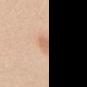  biopsy_status: not biopsied; imaged during a skin examination
  patient:
    sex: female
    age_approx: 20
  automated_metrics:
    eccentricity: 0.8
    shape_asymmetry: 0.3
  lesion_size:
    long_diameter_mm_approx: 3.5
  image:
    source: total-body photography crop
    field_of_view_mm: 15
  lighting: white-light
  site: mid back